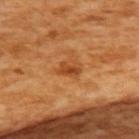biopsy status = catalogued during a skin exam; not biopsied
automated lesion analysis = a shape eccentricity near 0.75 and two-axis asymmetry of about 0.35; an average lesion color of about L≈49 a*≈28 b*≈44 (CIELAB) and roughly 10 lightness units darker than nearby skin; a border-irregularity index near 3/10, a color-variation rating of about 4/10, and peripheral color asymmetry of about 1.5; a nevus-likeness score of about 55/100 and lesion-presence confidence of about 100/100
patient = female, aged 53–57
body site = the upper back
imaging modality = ~15 mm crop, total-body skin-cancer survey
lesion diameter = ~2.5 mm (longest diameter)